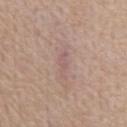Background:
From the front of the torso. A male patient approximately 75 years of age. Longest diameter approximately 3 mm. An algorithmic analysis of the crop reported a lesion area of about 3 mm², an outline eccentricity of about 0.9 (0 = round, 1 = elongated), and two-axis asymmetry of about 0.25. And it measured a nevus-likeness score of about 0/100. A 15 mm close-up tile from a total-body photography series done for melanoma screening. This is a white-light tile.